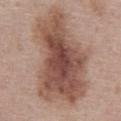The lesion was photographed on a routine skin check and not biopsied; there is no pathology result. Located on the front of the torso. A male subject roughly 60 years of age. A 15 mm close-up extracted from a 3D total-body photography capture.